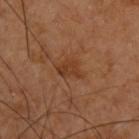{
  "biopsy_status": "not biopsied; imaged during a skin examination",
  "patient": {
    "sex": "male",
    "age_approx": 60
  },
  "image": {
    "source": "total-body photography crop",
    "field_of_view_mm": 15
  },
  "lesion_size": {
    "long_diameter_mm_approx": 3.0
  },
  "site": "upper back",
  "lighting": "cross-polarized"
}